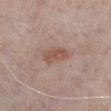{
  "biopsy_status": "not biopsied; imaged during a skin examination",
  "patient": {
    "sex": "male",
    "age_approx": 70
  },
  "site": "left lower leg",
  "image": {
    "source": "total-body photography crop",
    "field_of_view_mm": 15
  },
  "lesion_size": {
    "long_diameter_mm_approx": 4.0
  },
  "lighting": "white-light"
}